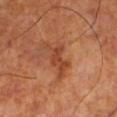Recorded during total-body skin imaging; not selected for excision or biopsy.
On the left lower leg.
Captured under cross-polarized illumination.
The total-body-photography lesion software estimated a shape-asymmetry score of about 0.55 (0 = symmetric). The analysis additionally found an average lesion color of about L≈42 a*≈27 b*≈34 (CIELAB), about 9 CIELAB-L* units darker than the surrounding skin, and a normalized border contrast of about 7. And it measured a border-irregularity rating of about 7/10 and a color-variation rating of about 2.5/10. And it measured an automated nevus-likeness rating near 0 out of 100 and a detector confidence of about 100 out of 100 that the crop contains a lesion.
A 15 mm close-up tile from a total-body photography series done for melanoma screening.
Measured at roughly 4.5 mm in maximum diameter.
A male patient, aged approximately 70.